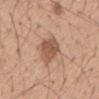{"biopsy_status": "not biopsied; imaged during a skin examination", "lesion_size": {"long_diameter_mm_approx": 5.0}, "patient": {"sex": "male", "age_approx": 55}, "site": "mid back", "image": {"source": "total-body photography crop", "field_of_view_mm": 15}, "lighting": "white-light"}